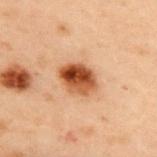biopsy status: no biopsy performed (imaged during a skin exam)
image: total-body-photography crop, ~15 mm field of view
tile lighting: cross-polarized illumination
patient: male, in their mid- to late 50s
automated metrics: a border-irregularity index near 1.5/10; lesion-presence confidence of about 100/100
body site: the upper back
diameter: ≈4 mm